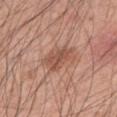follow-up: total-body-photography surveillance lesion; no biopsy | image source: total-body-photography crop, ~15 mm field of view | illumination: white-light illumination | automated lesion analysis: an outline eccentricity of about 0.7 (0 = round, 1 = elongated) and a shape-asymmetry score of about 0.3 (0 = symmetric); a mean CIELAB color near L≈52 a*≈23 b*≈28, a lesion–skin lightness drop of about 10, and a normalized lesion–skin contrast near 6.5; a border-irregularity index near 3.5/10, a color-variation rating of about 3/10, and a peripheral color-asymmetry measure near 1; a classifier nevus-likeness of about 10/100 and lesion-presence confidence of about 100/100 | patient: male, in their mid- to late 40s | anatomic site: the arm.